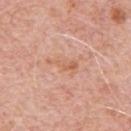  biopsy_status: not biopsied; imaged during a skin examination
  lesion_size:
    long_diameter_mm_approx: 3.5
  site: chest
  image:
    source: total-body photography crop
    field_of_view_mm: 15
  lighting: white-light
  patient:
    sex: male
    age_approx: 80
  automated_metrics:
    cielab_L: 62
    cielab_a: 23
    cielab_b: 33
    vs_skin_darker_L: 7.0
    vs_skin_contrast_norm: 5.5
    border_irregularity_0_10: 7.0
    color_variation_0_10: 2.5
    peripheral_color_asymmetry: 0.5
    lesion_detection_confidence_0_100: 100